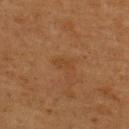Captured during whole-body skin photography for melanoma surveillance; the lesion was not biopsied.
Longest diameter approximately 2.5 mm.
A male patient in their mid-50s.
Located on the upper back.
Cropped from a whole-body photographic skin survey; the tile spans about 15 mm.
The total-body-photography lesion software estimated a lesion area of about 2.5 mm², an eccentricity of roughly 0.85, and a symmetry-axis asymmetry near 0.3. It also reported an average lesion color of about L≈37 a*≈20 b*≈32 (CIELAB). The analysis additionally found border irregularity of about 3 on a 0–10 scale, a within-lesion color-variation index near 0.5/10, and radial color variation of about 0.